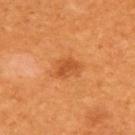The lesion was photographed on a routine skin check and not biopsied; there is no pathology result.
A 15 mm crop from a total-body photograph taken for skin-cancer surveillance.
Longest diameter approximately 3 mm.
Located on the upper back.
A male patient, aged approximately 60.
This is a cross-polarized tile.